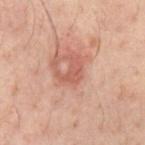<case>
<biopsy_status>not biopsied; imaged during a skin examination</biopsy_status>
<image>
  <source>total-body photography crop</source>
  <field_of_view_mm>15</field_of_view_mm>
</image>
<lighting>cross-polarized</lighting>
<site>chest</site>
<lesion_size>
  <long_diameter_mm_approx>2.5</long_diameter_mm_approx>
</lesion_size>
<patient>
  <sex>male</sex>
  <age_approx>40</age_approx>
</patient>
</case>